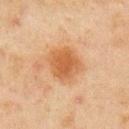<lesion>
<biopsy_status>not biopsied; imaged during a skin examination</biopsy_status>
<automated_metrics>
  <eccentricity>0.45</eccentricity>
  <shape_asymmetry>0.15</shape_asymmetry>
  <cielab_L>48</cielab_L>
  <cielab_a>20</cielab_a>
  <cielab_b>34</cielab_b>
  <border_irregularity_0_10>2.0</border_irregularity_0_10>
  <color_variation_0_10>3.0</color_variation_0_10>
  <peripheral_color_asymmetry>1.0</peripheral_color_asymmetry>
  <nevus_likeness_0_100>80</nevus_likeness_0_100>
  <lesion_detection_confidence_0_100>100</lesion_detection_confidence_0_100>
</automated_metrics>
<lesion_size>
  <long_diameter_mm_approx>4.5</long_diameter_mm_approx>
</lesion_size>
<image>
  <source>total-body photography crop</source>
  <field_of_view_mm>15</field_of_view_mm>
</image>
<patient>
  <sex>male</sex>
  <age_approx>45</age_approx>
</patient>
<site>arm</site>
</lesion>